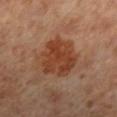biopsy status — no biopsy performed (imaged during a skin exam)
lesion size — ~6 mm (longest diameter)
image source — ~15 mm crop, total-body skin-cancer survey
lighting — cross-polarized
subject — female, aged approximately 55
image-analysis metrics — an average lesion color of about L≈42 a*≈25 b*≈34 (CIELAB), a lesion–skin lightness drop of about 10, and a lesion-to-skin contrast of about 9 (normalized; higher = more distinct); a border-irregularity rating of about 3/10 and internal color variation of about 3.5 on a 0–10 scale; a classifier nevus-likeness of about 80/100
location — the leg